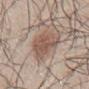Assessment:
Captured during whole-body skin photography for melanoma surveillance; the lesion was not biopsied.
Background:
Imaged with white-light lighting. This image is a 15 mm lesion crop taken from a total-body photograph. A male patient, in their mid-40s. The recorded lesion diameter is about 6 mm. The lesion is located on the back.